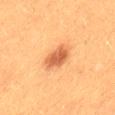Notes:
• workup: imaged on a skin check; not biopsied
• subject: female, aged 38–42
• tile lighting: cross-polarized illumination
• image source: total-body-photography crop, ~15 mm field of view
• location: the leg